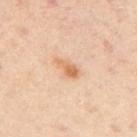workup: total-body-photography surveillance lesion; no biopsy | patient: male, aged approximately 55 | image source: 15 mm crop, total-body photography | anatomic site: the arm.